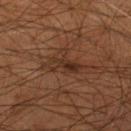Q: Is there a histopathology result?
A: no biopsy performed (imaged during a skin exam)
Q: How was this image acquired?
A: total-body-photography crop, ~15 mm field of view
Q: What is the anatomic site?
A: the right lower leg
Q: What are the patient's age and sex?
A: male, roughly 55 years of age